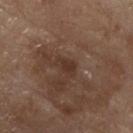notes: total-body-photography surveillance lesion; no biopsy
tile lighting: white-light illumination
lesion size: ≈2.5 mm
imaging modality: ~15 mm crop, total-body skin-cancer survey
location: the chest
patient: male, aged around 80